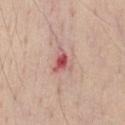Recorded during total-body skin imaging; not selected for excision or biopsy.
The lesion's longest dimension is about 3 mm.
Located on the chest.
A male subject, roughly 40 years of age.
A region of skin cropped from a whole-body photographic capture, roughly 15 mm wide.
The tile uses white-light illumination.